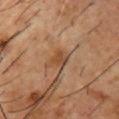| field | value |
|---|---|
| workup | imaged on a skin check; not biopsied |
| image source | 15 mm crop, total-body photography |
| site | the chest |
| lesion size | about 2.5 mm |
| image-analysis metrics | a border-irregularity index near 3/10, a color-variation rating of about 3/10, and a peripheral color-asymmetry measure near 1; a classifier nevus-likeness of about 5/100 and a detector confidence of about 100 out of 100 that the crop contains a lesion |
| subject | male, about 55 years old |